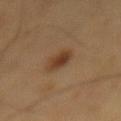<lesion>
<biopsy_status>not biopsied; imaged during a skin examination</biopsy_status>
<image>
  <source>total-body photography crop</source>
  <field_of_view_mm>15</field_of_view_mm>
</image>
<site>back</site>
<patient>
  <sex>male</sex>
  <age_approx>60</age_approx>
</patient>
</lesion>